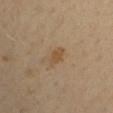Cropped from a total-body skin-imaging series; the visible field is about 15 mm.
A male subject roughly 55 years of age.
The tile uses cross-polarized illumination.
Measured at roughly 3 mm in maximum diameter.
The lesion is located on the arm.